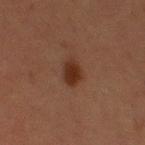Clinical impression: The lesion was tiled from a total-body skin photograph and was not biopsied. Clinical summary: A 15 mm close-up extracted from a 3D total-body photography capture. The patient is a female aged approximately 50. From the leg. Captured under cross-polarized illumination. The recorded lesion diameter is about 3.5 mm.